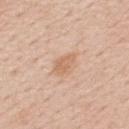notes: total-body-photography surveillance lesion; no biopsy | lighting: white-light illumination | patient: male, roughly 60 years of age | automated lesion analysis: a lesion color around L≈64 a*≈20 b*≈33 in CIELAB and about 8 CIELAB-L* units darker than the surrounding skin; internal color variation of about 1 on a 0–10 scale and radial color variation of about 0.5; a classifier nevus-likeness of about 20/100 | anatomic site: the mid back | imaging modality: 15 mm crop, total-body photography.